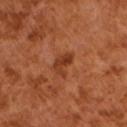<record>
  <biopsy_status>not biopsied; imaged during a skin examination</biopsy_status>
  <patient>
    <sex>male</sex>
    <age_approx>65</age_approx>
  </patient>
  <image>
    <source>total-body photography crop</source>
    <field_of_view_mm>15</field_of_view_mm>
  </image>
  <lesion_size>
    <long_diameter_mm_approx>3.0</long_diameter_mm_approx>
  </lesion_size>
  <lighting>cross-polarized</lighting>
  <automated_metrics>
    <area_mm2_approx>5.0</area_mm2_approx>
    <eccentricity>0.8</eccentricity>
    <cielab_L>37</cielab_L>
    <cielab_a>27</cielab_a>
    <cielab_b>35</cielab_b>
    <vs_skin_darker_L>9.0</vs_skin_darker_L>
    <vs_skin_contrast_norm>7.5</vs_skin_contrast_norm>
    <border_irregularity_0_10>4.0</border_irregularity_0_10>
    <color_variation_0_10>3.5</color_variation_0_10>
    <peripheral_color_asymmetry>1.5</peripheral_color_asymmetry>
    <nevus_likeness_0_100>0</nevus_likeness_0_100>
    <lesion_detection_confidence_0_100>100</lesion_detection_confidence_0_100>
  </automated_metrics>
</record>